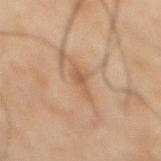notes: catalogued during a skin exam; not biopsied | subject: male, aged approximately 50 | size: ≈2.5 mm | location: the abdomen | image-analysis metrics: an area of roughly 3 mm² and a symmetry-axis asymmetry near 0.4; a border-irregularity rating of about 4/10 and a within-lesion color-variation index near 1/10; an automated nevus-likeness rating near 0 out of 100 | image source: total-body-photography crop, ~15 mm field of view | lighting: cross-polarized.